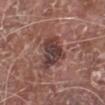{
  "biopsy_status": "not biopsied; imaged during a skin examination",
  "lighting": "white-light",
  "image": {
    "source": "total-body photography crop",
    "field_of_view_mm": 15
  },
  "patient": {
    "sex": "male",
    "age_approx": 80
  },
  "lesion_size": {
    "long_diameter_mm_approx": 4.5
  },
  "site": "leg"
}